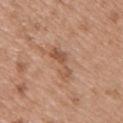| key | value |
|---|---|
| follow-up | total-body-photography surveillance lesion; no biopsy |
| patient | male, about 50 years old |
| size | ≈4.5 mm |
| tile lighting | white-light |
| imaging modality | ~15 mm tile from a whole-body skin photo |
| location | the back |